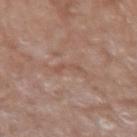<tbp_lesion>
<biopsy_status>not biopsied; imaged during a skin examination</biopsy_status>
<site>arm</site>
<image>
  <source>total-body photography crop</source>
  <field_of_view_mm>15</field_of_view_mm>
</image>
<patient>
  <sex>female</sex>
  <age_approx>75</age_approx>
</patient>
<lighting>white-light</lighting>
</tbp_lesion>